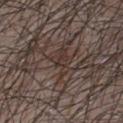Clinical impression:
Captured during whole-body skin photography for melanoma surveillance; the lesion was not biopsied.
Acquisition and patient details:
A male patient, roughly 40 years of age. This image is a 15 mm lesion crop taken from a total-body photograph. The lesion is located on the chest.